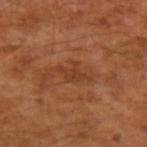No biopsy was performed on this lesion — it was imaged during a full skin examination and was not determined to be concerning.
Captured under cross-polarized illumination.
A lesion tile, about 15 mm wide, cut from a 3D total-body photograph.
The lesion is located on the left upper arm.
A male patient aged around 55.